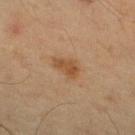The lesion is on the right lower leg. The patient is a male aged around 70. Longest diameter approximately 3.5 mm. Automated tile analysis of the lesion measured a border-irregularity index near 2.5/10, internal color variation of about 2 on a 0–10 scale, and peripheral color asymmetry of about 0.5. And it measured a nevus-likeness score of about 80/100. A lesion tile, about 15 mm wide, cut from a 3D total-body photograph.